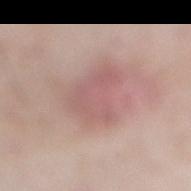biopsy_status: not biopsied; imaged during a skin examination
patient:
  sex: male
  age_approx: 60
image:
  source: total-body photography crop
  field_of_view_mm: 15
lighting: white-light
automated_metrics:
  area_mm2_approx: 13.0
  eccentricity: 0.8
  shape_asymmetry: 0.4
  border_irregularity_0_10: 4.5
  peripheral_color_asymmetry: 1.0
  nevus_likeness_0_100: 55
  lesion_detection_confidence_0_100: 100
site: right lower leg